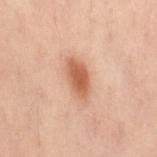No biopsy was performed on this lesion — it was imaged during a full skin examination and was not determined to be concerning. On the lower back. A female patient aged 53–57. A lesion tile, about 15 mm wide, cut from a 3D total-body photograph. The tile uses cross-polarized illumination. Automated image analysis of the tile measured an area of roughly 8 mm², an eccentricity of roughly 0.8, and a symmetry-axis asymmetry near 0.2. It also reported border irregularity of about 2.5 on a 0–10 scale, a color-variation rating of about 2.5/10, and radial color variation of about 0.5. The software also gave an automated nevus-likeness rating near 95 out of 100 and a detector confidence of about 100 out of 100 that the crop contains a lesion.